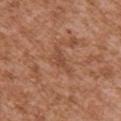Q: Was a biopsy performed?
A: no biopsy performed (imaged during a skin exam)
Q: What is the anatomic site?
A: the chest
Q: How large is the lesion?
A: ≈3.5 mm
Q: Illumination type?
A: white-light
Q: Who is the patient?
A: male, roughly 45 years of age
Q: How was this image acquired?
A: ~15 mm crop, total-body skin-cancer survey